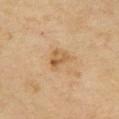follow-up = imaged on a skin check; not biopsied | TBP lesion metrics = a lesion area of about 5.5 mm², a shape eccentricity near 0.6, and a symmetry-axis asymmetry near 0.35; an average lesion color of about L≈55 a*≈17 b*≈37 (CIELAB), a lesion–skin lightness drop of about 8, and a normalized border contrast of about 6.5; a border-irregularity rating of about 3.5/10 and peripheral color asymmetry of about 2 | subject = male, about 70 years old | body site = the chest | image = 15 mm crop, total-body photography | lesion size = ~3 mm (longest diameter).